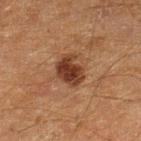Notes:
- notes · imaged on a skin check; not biopsied
- image source · total-body-photography crop, ~15 mm field of view
- subject · male, aged 58 to 62
- site · the left lower leg
- automated metrics · a lesion color around L≈29 a*≈18 b*≈25 in CIELAB, about 11 CIELAB-L* units darker than the surrounding skin, and a normalized lesion–skin contrast near 10.5; a classifier nevus-likeness of about 80/100 and lesion-presence confidence of about 100/100
- size · about 4 mm